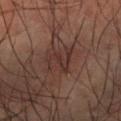The lesion was photographed on a routine skin check and not biopsied; there is no pathology result.
The lesion is located on the arm.
Cropped from a whole-body photographic skin survey; the tile spans about 15 mm.
Approximately 4 mm at its widest.
The subject is a male about 50 years old.